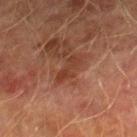Clinical impression: Part of a total-body skin-imaging series; this lesion was reviewed on a skin check and was not flagged for biopsy. Context: A male subject aged around 75. An algorithmic analysis of the crop reported a lesion area of about 3.5 mm², a shape eccentricity near 0.95, and a shape-asymmetry score of about 0.35 (0 = symmetric). The analysis additionally found a lesion–skin lightness drop of about 7 and a normalized lesion–skin contrast near 7. It also reported a nevus-likeness score of about 0/100. On the leg. A 15 mm crop from a total-body photograph taken for skin-cancer surveillance. About 3.5 mm across.